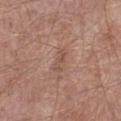This image is a 15 mm lesion crop taken from a total-body photograph. Imaged with white-light lighting. An algorithmic analysis of the crop reported a lesion area of about 4 mm², an outline eccentricity of about 0.9 (0 = round, 1 = elongated), and a shape-asymmetry score of about 0.2 (0 = symmetric). It also reported border irregularity of about 2.5 on a 0–10 scale and radial color variation of about 0.5. Located on the leg. Longest diameter approximately 3 mm. The patient is a male aged 53–57.